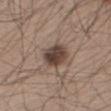– workup — total-body-photography surveillance lesion; no biopsy